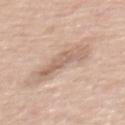| feature | finding |
|---|---|
| follow-up | total-body-photography surveillance lesion; no biopsy |
| size | ≈5.5 mm |
| image-analysis metrics | border irregularity of about 4.5 on a 0–10 scale, internal color variation of about 4 on a 0–10 scale, and radial color variation of about 1.5; an automated nevus-likeness rating near 0 out of 100 |
| body site | the back |
| patient | male, aged approximately 60 |
| illumination | white-light illumination |
| acquisition | ~15 mm crop, total-body skin-cancer survey |